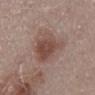location=the mid back | patient=male, aged 53–57 | image=15 mm crop, total-body photography | diameter=about 7.5 mm.